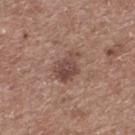Q: Was a biopsy performed?
A: no biopsy performed (imaged during a skin exam)
Q: Who is the patient?
A: male, aged approximately 60
Q: How was this image acquired?
A: ~15 mm crop, total-body skin-cancer survey
Q: Where on the body is the lesion?
A: the upper back